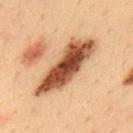Impression: The lesion was tiled from a total-body skin photograph and was not biopsied. Context: A male subject, roughly 35 years of age. The lesion is located on the upper back. Imaged with cross-polarized lighting. A roughly 15 mm field-of-view crop from a total-body skin photograph.